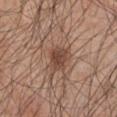Q: Was this lesion biopsied?
A: total-body-photography surveillance lesion; no biopsy
Q: What did automated image analysis measure?
A: a lesion area of about 7 mm², an outline eccentricity of about 0.6 (0 = round, 1 = elongated), and a shape-asymmetry score of about 0.3 (0 = symmetric); about 10 CIELAB-L* units darker than the surrounding skin and a lesion-to-skin contrast of about 8 (normalized; higher = more distinct); a classifier nevus-likeness of about 80/100 and a detector confidence of about 100 out of 100 that the crop contains a lesion
Q: Illumination type?
A: white-light
Q: What is the imaging modality?
A: 15 mm crop, total-body photography
Q: What is the anatomic site?
A: the arm
Q: Patient demographics?
A: male, aged 43–47